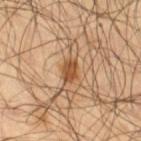Impression:
Recorded during total-body skin imaging; not selected for excision or biopsy.
Context:
A close-up tile cropped from a whole-body skin photograph, about 15 mm across. Located on the right thigh. The subject is a male aged 53–57.